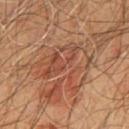Clinical impression:
Captured during whole-body skin photography for melanoma surveillance; the lesion was not biopsied.
Image and clinical context:
A region of skin cropped from a whole-body photographic capture, roughly 15 mm wide. About 6.5 mm across. The tile uses cross-polarized illumination. Automated tile analysis of the lesion measured a lesion area of about 22 mm², an outline eccentricity of about 0.5 (0 = round, 1 = elongated), and a symmetry-axis asymmetry near 0.45. It also reported a lesion color around L≈44 a*≈22 b*≈29 in CIELAB, a lesion–skin lightness drop of about 7, and a normalized lesion–skin contrast near 6. The software also gave a nevus-likeness score of about 15/100 and a lesion-detection confidence of about 95/100. The lesion is located on the chest. The subject is a male in their 60s.